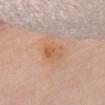{
  "biopsy_status": "not biopsied; imaged during a skin examination",
  "patient": {
    "sex": "female",
    "age_approx": "85+"
  },
  "image": {
    "source": "total-body photography crop",
    "field_of_view_mm": 15
  },
  "site": "chest",
  "lesion_size": {
    "long_diameter_mm_approx": 3.0
  },
  "lighting": "white-light"
}